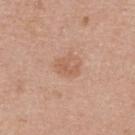{"biopsy_status": "not biopsied; imaged during a skin examination", "lighting": "white-light", "patient": {"sex": "female", "age_approx": 40}, "site": "upper back", "image": {"source": "total-body photography crop", "field_of_view_mm": 15}, "lesion_size": {"long_diameter_mm_approx": 2.5}}